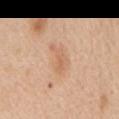Imaged during a routine full-body skin examination; the lesion was not biopsied and no histopathology is available. Captured under white-light illumination. Automated image analysis of the tile measured a lesion area of about 5.5 mm², an outline eccentricity of about 0.9 (0 = round, 1 = elongated), and two-axis asymmetry of about 0.5. The analysis additionally found a within-lesion color-variation index near 2.5/10 and radial color variation of about 1. And it measured a nevus-likeness score of about 0/100. A male patient roughly 60 years of age. A 15 mm crop from a total-body photograph taken for skin-cancer surveillance. From the mid back.